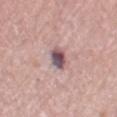| field | value |
|---|---|
| image | total-body-photography crop, ~15 mm field of view |
| patient | male, roughly 80 years of age |
| size | ≈3 mm |
| tile lighting | white-light |
| body site | the right thigh |